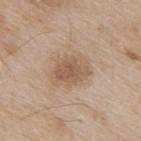Clinical impression: This lesion was catalogued during total-body skin photography and was not selected for biopsy. Acquisition and patient details: A male subject aged approximately 65. A 15 mm crop from a total-body photograph taken for skin-cancer surveillance. Longest diameter approximately 4.5 mm. The lesion is located on the back.